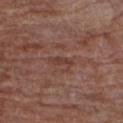Recorded during total-body skin imaging; not selected for excision or biopsy. A male subject in their 70s. About 3 mm across. A 15 mm crop from a total-body photograph taken for skin-cancer surveillance. The lesion is located on the right forearm.